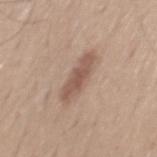This lesion was catalogued during total-body skin photography and was not selected for biopsy. The lesion-visualizer software estimated a footprint of about 8.5 mm² and an outline eccentricity of about 0.95 (0 = round, 1 = elongated). The software also gave a border-irregularity rating of about 3.5/10, internal color variation of about 2 on a 0–10 scale, and peripheral color asymmetry of about 0.5. And it measured an automated nevus-likeness rating near 45 out of 100. Approximately 5.5 mm at its widest. Cropped from a total-body skin-imaging series; the visible field is about 15 mm. Located on the back. A male subject roughly 40 years of age.